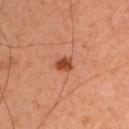notes: imaged on a skin check; not biopsied
patient: male, aged 43–47
size: ≈2.5 mm
body site: the left upper arm
image source: ~15 mm tile from a whole-body skin photo
illumination: cross-polarized illumination